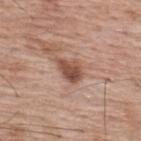No biopsy was performed on this lesion — it was imaged during a full skin examination and was not determined to be concerning. An algorithmic analysis of the crop reported two-axis asymmetry of about 0.3. And it measured an average lesion color of about L≈50 a*≈22 b*≈27 (CIELAB) and about 13 CIELAB-L* units darker than the surrounding skin. It also reported a nevus-likeness score of about 50/100 and lesion-presence confidence of about 100/100. A male subject aged around 70. Imaged with white-light lighting. A close-up tile cropped from a whole-body skin photograph, about 15 mm across. Located on the upper back.